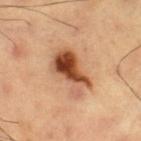{
  "biopsy_status": "not biopsied; imaged during a skin examination",
  "lesion_size": {
    "long_diameter_mm_approx": 6.0
  },
  "automated_metrics": {
    "area_mm2_approx": 15.0,
    "eccentricity": 0.85,
    "shape_asymmetry": 0.3,
    "border_irregularity_0_10": 4.0,
    "peripheral_color_asymmetry": 4.0,
    "lesion_detection_confidence_0_100": 100
  },
  "lighting": "cross-polarized",
  "patient": {
    "sex": "male",
    "age_approx": 60
  },
  "site": "leg",
  "image": {
    "source": "total-body photography crop",
    "field_of_view_mm": 15
  }
}